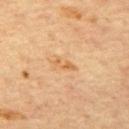<case>
<biopsy_status>not biopsied; imaged during a skin examination</biopsy_status>
<image>
  <source>total-body photography crop</source>
  <field_of_view_mm>15</field_of_view_mm>
</image>
<site>upper back</site>
<patient>
  <sex>male</sex>
  <age_approx>65</age_approx>
</patient>
</case>